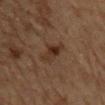Recorded during total-body skin imaging; not selected for excision or biopsy. On the mid back. A 15 mm crop from a total-body photograph taken for skin-cancer surveillance. Captured under cross-polarized illumination. The lesion-visualizer software estimated an outline eccentricity of about 0.65 (0 = round, 1 = elongated) and a symmetry-axis asymmetry near 0.35. The software also gave an average lesion color of about L≈26 a*≈15 b*≈23 (CIELAB), a lesion–skin lightness drop of about 7, and a normalized lesion–skin contrast near 8. The analysis additionally found radial color variation of about 2. And it measured a classifier nevus-likeness of about 65/100 and a lesion-detection confidence of about 100/100. A male subject, aged 83 or over. Measured at roughly 2.5 mm in maximum diameter.